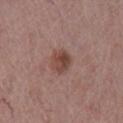Notes:
* follow-up: no biopsy performed (imaged during a skin exam)
* automated lesion analysis: an area of roughly 6 mm² and a symmetry-axis asymmetry near 0.15; an average lesion color of about L≈44 a*≈21 b*≈24 (CIELAB), a lesion–skin lightness drop of about 10, and a normalized lesion–skin contrast near 8
* subject: female, in their mid-60s
* lesion size: ~2.5 mm (longest diameter)
* image source: total-body-photography crop, ~15 mm field of view
* anatomic site: the left lower leg